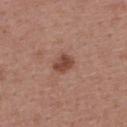• follow-up: catalogued during a skin exam; not biopsied
• image source: ~15 mm crop, total-body skin-cancer survey
• patient: female, in their 50s
• site: the upper back